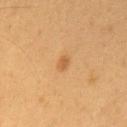<lesion>
  <biopsy_status>not biopsied; imaged during a skin examination</biopsy_status>
  <lighting>cross-polarized</lighting>
  <image>
    <source>total-body photography crop</source>
    <field_of_view_mm>15</field_of_view_mm>
  </image>
  <patient>
    <sex>male</sex>
    <age_approx>60</age_approx>
  </patient>
  <lesion_size>
    <long_diameter_mm_approx>2.0</long_diameter_mm_approx>
  </lesion_size>
  <site>chest</site>
  <automated_metrics>
    <area_mm2_approx>2.5</area_mm2_approx>
    <shape_asymmetry>0.3</shape_asymmetry>
    <cielab_L>55</cielab_L>
    <cielab_a>20</cielab_a>
    <cielab_b>41</cielab_b>
    <vs_skin_darker_L>8.0</vs_skin_darker_L>
    <vs_skin_contrast_norm>6.0</vs_skin_contrast_norm>
    <border_irregularity_0_10>2.5</border_irregularity_0_10>
    <color_variation_0_10>1.5</color_variation_0_10>
    <peripheral_color_asymmetry>0.5</peripheral_color_asymmetry>
  </automated_metrics>
</lesion>